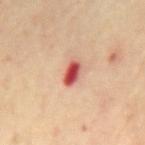Clinical impression: The lesion was photographed on a routine skin check and not biopsied; there is no pathology result. Context: A male subject, aged 68–72. From the mid back. Measured at roughly 3 mm in maximum diameter. This is a cross-polarized tile. A region of skin cropped from a whole-body photographic capture, roughly 15 mm wide. The total-body-photography lesion software estimated a shape eccentricity near 0.8 and a shape-asymmetry score of about 0.25 (0 = symmetric). The software also gave an average lesion color of about L≈53 a*≈34 b*≈29 (CIELAB), a lesion–skin lightness drop of about 18, and a lesion-to-skin contrast of about 11.5 (normalized; higher = more distinct). And it measured lesion-presence confidence of about 100/100.